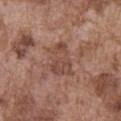Notes:
- follow-up: no biopsy performed (imaged during a skin exam)
- imaging modality: ~15 mm crop, total-body skin-cancer survey
- lesion diameter: ~4.5 mm (longest diameter)
- body site: the abdomen
- tile lighting: white-light illumination
- patient: male, aged 73 to 77